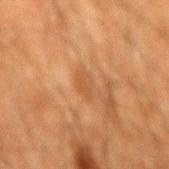Impression:
Recorded during total-body skin imaging; not selected for excision or biopsy.
Clinical summary:
Cropped from a total-body skin-imaging series; the visible field is about 15 mm. The lesion is located on the mid back. A male subject, roughly 60 years of age.